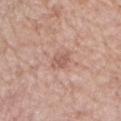Assessment:
The lesion was photographed on a routine skin check and not biopsied; there is no pathology result.
Image and clinical context:
Imaged with white-light lighting. The lesion is on the arm. Cropped from a whole-body photographic skin survey; the tile spans about 15 mm. A female patient, aged approximately 75.